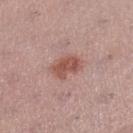Impression:
The lesion was photographed on a routine skin check and not biopsied; there is no pathology result.
Clinical summary:
A lesion tile, about 15 mm wide, cut from a 3D total-body photograph. On the left lower leg. A female patient in their mid- to late 50s. An algorithmic analysis of the crop reported an average lesion color of about L≈53 a*≈23 b*≈25 (CIELAB), a lesion–skin lightness drop of about 10, and a normalized lesion–skin contrast near 7.5. And it measured a border-irregularity index near 2.5/10 and internal color variation of about 3.5 on a 0–10 scale. This is a white-light tile. Approximately 4 mm at its widest.